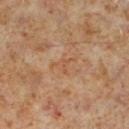Imaged during a routine full-body skin examination; the lesion was not biopsied and no histopathology is available.
An algorithmic analysis of the crop reported a lesion area of about 3 mm² and a symmetry-axis asymmetry near 0.65. The software also gave a border-irregularity rating of about 7/10. It also reported a nevus-likeness score of about 0/100.
On the left lower leg.
The tile uses cross-polarized illumination.
Longest diameter approximately 3 mm.
A 15 mm close-up extracted from a 3D total-body photography capture.
A male subject roughly 60 years of age.